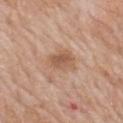Notes:
* notes — no biopsy performed (imaged during a skin exam)
* image — ~15 mm tile from a whole-body skin photo
* diameter — ≈4 mm
* TBP lesion metrics — border irregularity of about 2.5 on a 0–10 scale and radial color variation of about 1; a classifier nevus-likeness of about 10/100 and a detector confidence of about 100 out of 100 that the crop contains a lesion
* location — the back
* patient — male, in their 60s